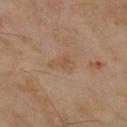Impression:
Part of a total-body skin-imaging series; this lesion was reviewed on a skin check and was not flagged for biopsy.
Clinical summary:
The total-body-photography lesion software estimated a lesion area of about 5 mm², a shape eccentricity near 0.8, and a shape-asymmetry score of about 0.45 (0 = symmetric). The analysis additionally found a mean CIELAB color near L≈47 a*≈15 b*≈29, roughly 5 lightness units darker than nearby skin, and a normalized lesion–skin contrast near 5. It also reported border irregularity of about 4 on a 0–10 scale, a color-variation rating of about 1.5/10, and a peripheral color-asymmetry measure near 0.5. A region of skin cropped from a whole-body photographic capture, roughly 15 mm wide. The lesion is located on the right lower leg. This is a cross-polarized tile. About 3.5 mm across. The subject is a female aged 58–62.